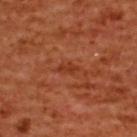Q: Was a biopsy performed?
A: total-body-photography surveillance lesion; no biopsy
Q: Lesion size?
A: about 2.5 mm
Q: Who is the patient?
A: female, in their mid- to late 50s
Q: What kind of image is this?
A: ~15 mm tile from a whole-body skin photo
Q: What is the anatomic site?
A: the upper back
Q: What did automated image analysis measure?
A: a mean CIELAB color near L≈39 a*≈32 b*≈38, roughly 7 lightness units darker than nearby skin, and a lesion-to-skin contrast of about 6.5 (normalized; higher = more distinct); a nevus-likeness score of about 0/100 and a lesion-detection confidence of about 100/100
Q: What lighting was used for the tile?
A: cross-polarized